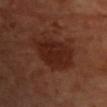Q: Was this lesion biopsied?
A: no biopsy performed (imaged during a skin exam)
Q: What is the anatomic site?
A: the head or neck
Q: What kind of image is this?
A: total-body-photography crop, ~15 mm field of view
Q: What did automated image analysis measure?
A: a lesion area of about 17 mm², an eccentricity of roughly 0.65, and two-axis asymmetry of about 0.25
Q: Illumination type?
A: cross-polarized illumination
Q: What are the patient's age and sex?
A: male, aged approximately 65